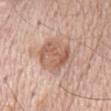follow-up = total-body-photography surveillance lesion; no biopsy | acquisition = ~15 mm crop, total-body skin-cancer survey | body site = the chest | patient = male, aged 68 to 72.